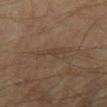Imaged during a routine full-body skin examination; the lesion was not biopsied and no histopathology is available.
The recorded lesion diameter is about 4 mm.
The patient is a male aged around 70.
On the right thigh.
A 15 mm close-up extracted from a 3D total-body photography capture.
The total-body-photography lesion software estimated a lesion area of about 5 mm² and two-axis asymmetry of about 0.5.
Imaged with cross-polarized lighting.